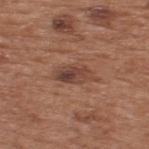Recorded during total-body skin imaging; not selected for excision or biopsy.
The patient is a male roughly 55 years of age.
A close-up tile cropped from a whole-body skin photograph, about 15 mm across.
Approximately 4.5 mm at its widest.
On the upper back.